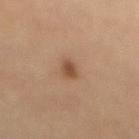<case>
<biopsy_status>not biopsied; imaged during a skin examination</biopsy_status>
<patient>
  <sex>male</sex>
  <age_approx>60</age_approx>
</patient>
<lesion_size>
  <long_diameter_mm_approx>2.5</long_diameter_mm_approx>
</lesion_size>
<site>mid back</site>
<lighting>cross-polarized</lighting>
<automated_metrics>
  <area_mm2_approx>3.0</area_mm2_approx>
  <eccentricity>0.8</eccentricity>
  <cielab_L>47</cielab_L>
  <cielab_a>19</cielab_a>
  <cielab_b>31</cielab_b>
  <vs_skin_darker_L>10.0</vs_skin_darker_L>
  <vs_skin_contrast_norm>8.0</vs_skin_contrast_norm>
  <border_irregularity_0_10>2.5</border_irregularity_0_10>
  <color_variation_0_10>0.5</color_variation_0_10>
  <peripheral_color_asymmetry>0.0</peripheral_color_asymmetry>
  <nevus_likeness_0_100>95</nevus_likeness_0_100>
  <lesion_detection_confidence_0_100>100</lesion_detection_confidence_0_100>
</automated_metrics>
<image>
  <source>total-body photography crop</source>
  <field_of_view_mm>15</field_of_view_mm>
</image>
</case>